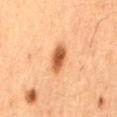Clinical impression:
No biopsy was performed on this lesion — it was imaged during a full skin examination and was not determined to be concerning.
Acquisition and patient details:
The lesion-visualizer software estimated a mean CIELAB color near L≈49 a*≈23 b*≈36, about 14 CIELAB-L* units darker than the surrounding skin, and a lesion-to-skin contrast of about 10 (normalized; higher = more distinct). And it measured a border-irregularity rating of about 2/10 and peripheral color asymmetry of about 1. Longest diameter approximately 4 mm. The patient is a male in their mid- to late 60s. Imaged with cross-polarized lighting. The lesion is located on the mid back. A roughly 15 mm field-of-view crop from a total-body skin photograph.